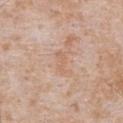biopsy_status: not biopsied; imaged during a skin examination
image:
  source: total-body photography crop
  field_of_view_mm: 15
lighting: white-light
patient:
  sex: male
  age_approx: 65
site: abdomen